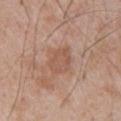follow-up: catalogued during a skin exam; not biopsied
anatomic site: the chest
acquisition: 15 mm crop, total-body photography
image-analysis metrics: an area of roughly 8 mm², an outline eccentricity of about 0.5 (0 = round, 1 = elongated), and a shape-asymmetry score of about 0.2 (0 = symmetric); a mean CIELAB color near L≈55 a*≈21 b*≈29 and a lesion-to-skin contrast of about 5 (normalized; higher = more distinct); a within-lesion color-variation index near 2.5/10 and radial color variation of about 0.5; an automated nevus-likeness rating near 0 out of 100 and a lesion-detection confidence of about 100/100
subject: male, about 65 years old
illumination: white-light